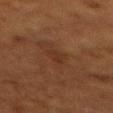Clinical impression:
Captured during whole-body skin photography for melanoma surveillance; the lesion was not biopsied.
Image and clinical context:
The patient is a female roughly 50 years of age. On the mid back. Captured under cross-polarized illumination. Approximately 3 mm at its widest. Cropped from a total-body skin-imaging series; the visible field is about 15 mm.